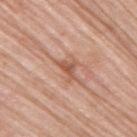biopsy_status: not biopsied; imaged during a skin examination
automated_metrics:
  cielab_L: 56
  cielab_a: 24
  cielab_b: 31
  vs_skin_darker_L: 10.0
  color_variation_0_10: 1.5
  peripheral_color_asymmetry: 0.5
site: right upper arm
lighting: white-light
patient:
  sex: male
  age_approx: 80
image:
  source: total-body photography crop
  field_of_view_mm: 15